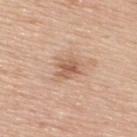workup: no biopsy performed (imaged during a skin exam) | imaging modality: ~15 mm tile from a whole-body skin photo | lesion size: ≈2.5 mm | tile lighting: white-light illumination | patient: male, about 50 years old | anatomic site: the upper back | automated metrics: an eccentricity of roughly 0.55 and a shape-asymmetry score of about 0.3 (0 = symmetric); a nevus-likeness score of about 20/100 and a detector confidence of about 100 out of 100 that the crop contains a lesion.